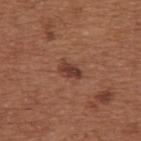The lesion was tiled from a total-body skin photograph and was not biopsied.
A 15 mm crop from a total-body photograph taken for skin-cancer surveillance.
An algorithmic analysis of the crop reported an area of roughly 4 mm², an eccentricity of roughly 0.8, and a symmetry-axis asymmetry near 0.3. The analysis additionally found a border-irregularity rating of about 3/10, a color-variation rating of about 1.5/10, and peripheral color asymmetry of about 0.5. The software also gave a nevus-likeness score of about 90/100.
The tile uses white-light illumination.
A female patient, in their mid-60s.
Approximately 3 mm at its widest.
The lesion is on the upper back.